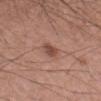Clinical impression:
The lesion was tiled from a total-body skin photograph and was not biopsied.
Acquisition and patient details:
A close-up tile cropped from a whole-body skin photograph, about 15 mm across. The lesion is on the left forearm. A male subject, in their mid- to late 50s.